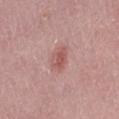biopsy_status: not biopsied; imaged during a skin examination
image:
  source: total-body photography crop
  field_of_view_mm: 15
lesion_size:
  long_diameter_mm_approx: 2.5
site: right thigh
lighting: white-light
automated_metrics:
  eccentricity: 0.65
  shape_asymmetry: 0.2
  vs_skin_darker_L: 9.0
  vs_skin_contrast_norm: 6.5
patient:
  sex: female
  age_approx: 45